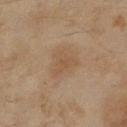Part of a total-body skin-imaging series; this lesion was reviewed on a skin check and was not flagged for biopsy. Measured at roughly 3.5 mm in maximum diameter. From the left lower leg. A female subject, roughly 60 years of age. A lesion tile, about 15 mm wide, cut from a 3D total-body photograph. The tile uses cross-polarized illumination. The total-body-photography lesion software estimated a footprint of about 5.5 mm², a shape eccentricity near 0.65, and a symmetry-axis asymmetry near 0.55. It also reported border irregularity of about 5 on a 0–10 scale, a color-variation rating of about 1.5/10, and radial color variation of about 0.5. The software also gave a classifier nevus-likeness of about 0/100.